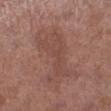No biopsy was performed on this lesion — it was imaged during a full skin examination and was not determined to be concerning. Longest diameter approximately 6.5 mm. This is a white-light tile. A 15 mm crop from a total-body photograph taken for skin-cancer surveillance. The patient is a female aged 48–52. The lesion is on the left lower leg.